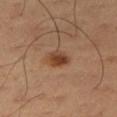Impression:
This lesion was catalogued during total-body skin photography and was not selected for biopsy.
Clinical summary:
A male subject, aged around 50. Located on the right thigh. A region of skin cropped from a whole-body photographic capture, roughly 15 mm wide. This is a cross-polarized tile.